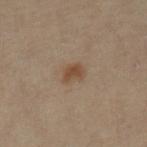Part of a total-body skin-imaging series; this lesion was reviewed on a skin check and was not flagged for biopsy. Imaged with cross-polarized lighting. A female subject, about 50 years old. A close-up tile cropped from a whole-body skin photograph, about 15 mm across. The lesion-visualizer software estimated radial color variation of about 0.5. About 3 mm across. From the left lower leg.